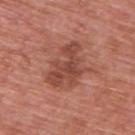Notes:
- workup: total-body-photography surveillance lesion; no biopsy
- image source: 15 mm crop, total-body photography
- subject: male, about 70 years old
- diameter: about 5.5 mm
- body site: the upper back
- illumination: white-light